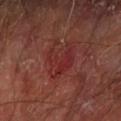Clinical summary:
Captured under cross-polarized illumination. The lesion is located on the left forearm. Approximately 4 mm at its widest. A 15 mm crop from a total-body photograph taken for skin-cancer surveillance. The subject is a male in their 70s.